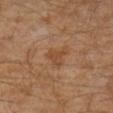follow-up: catalogued during a skin exam; not biopsied | illumination: cross-polarized | lesion diameter: about 3 mm | image: ~15 mm crop, total-body skin-cancer survey | anatomic site: the leg | TBP lesion metrics: a border-irregularity rating of about 5.5/10, a color-variation rating of about 1.5/10, and a peripheral color-asymmetry measure near 0.5; an automated nevus-likeness rating near 0 out of 100 and a lesion-detection confidence of about 100/100 | subject: male, aged 28 to 32.